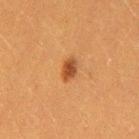Assessment: No biopsy was performed on this lesion — it was imaged during a full skin examination and was not determined to be concerning. Background: On the left thigh. Imaged with cross-polarized lighting. The patient is a female aged 38–42. A lesion tile, about 15 mm wide, cut from a 3D total-body photograph. Automated tile analysis of the lesion measured roughly 11 lightness units darker than nearby skin and a lesion-to-skin contrast of about 8.5 (normalized; higher = more distinct). The software also gave a lesion-detection confidence of about 100/100. The recorded lesion diameter is about 3 mm.